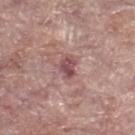Q: Was this lesion biopsied?
A: no biopsy performed (imaged during a skin exam)
Q: How was the tile lit?
A: white-light illumination
Q: What is the lesion's diameter?
A: ≈2.5 mm
Q: What is the imaging modality?
A: total-body-photography crop, ~15 mm field of view
Q: What are the patient's age and sex?
A: female, aged approximately 60
Q: Lesion location?
A: the leg